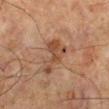Q: Where on the body is the lesion?
A: the right lower leg
Q: Patient demographics?
A: male, aged 63–67
Q: How was this image acquired?
A: ~15 mm tile from a whole-body skin photo